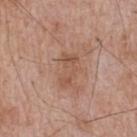Q: Was a biopsy performed?
A: no biopsy performed (imaged during a skin exam)
Q: What did automated image analysis measure?
A: an average lesion color of about L≈52 a*≈21 b*≈30 (CIELAB) and a lesion-to-skin contrast of about 6 (normalized; higher = more distinct); border irregularity of about 7 on a 0–10 scale and internal color variation of about 2 on a 0–10 scale; an automated nevus-likeness rating near 0 out of 100
Q: Who is the patient?
A: male, aged approximately 65
Q: Illumination type?
A: white-light illumination
Q: How was this image acquired?
A: total-body-photography crop, ~15 mm field of view
Q: Where on the body is the lesion?
A: the upper back